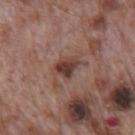Clinical impression: No biopsy was performed on this lesion — it was imaged during a full skin examination and was not determined to be concerning. Image and clinical context: About 3 mm across. A roughly 15 mm field-of-view crop from a total-body skin photograph. The tile uses white-light illumination. An algorithmic analysis of the crop reported a footprint of about 6 mm² and a shape eccentricity near 0.7. The analysis additionally found an average lesion color of about L≈39 a*≈20 b*≈22 (CIELAB). The subject is a male approximately 70 years of age. The lesion is located on the mid back.